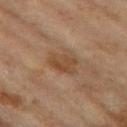Impression: Part of a total-body skin-imaging series; this lesion was reviewed on a skin check and was not flagged for biopsy. Image and clinical context: About 3.5 mm across. The patient is a female in their 60s. From the left thigh. Imaged with cross-polarized lighting. A lesion tile, about 15 mm wide, cut from a 3D total-body photograph.